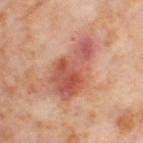The lesion was tiled from a total-body skin photograph and was not biopsied.
A region of skin cropped from a whole-body photographic capture, roughly 15 mm wide.
A female subject, aged 53 to 57.
The lesion is on the right thigh.